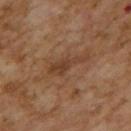workup: catalogued during a skin exam; not biopsied
body site: the upper back
image: ~15 mm tile from a whole-body skin photo
lighting: cross-polarized
patient: female, aged 58 to 62
automated metrics: an area of roughly 6 mm², a shape eccentricity near 0.95, and a symmetry-axis asymmetry near 0.45; an average lesion color of about L≈34 a*≈17 b*≈28 (CIELAB), a lesion–skin lightness drop of about 7, and a normalized lesion–skin contrast near 6.5